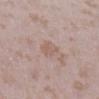The lesion was tiled from a total-body skin photograph and was not biopsied. The recorded lesion diameter is about 2.5 mm. A female patient in their mid- to late 20s. This is a white-light tile. From the right lower leg. A region of skin cropped from a whole-body photographic capture, roughly 15 mm wide. The total-body-photography lesion software estimated an area of roughly 4.5 mm², an eccentricity of roughly 0.7, and two-axis asymmetry of about 0.25.